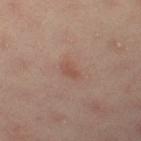The lesion was tiled from a total-body skin photograph and was not biopsied.
The subject is a female aged 53–57.
This is a cross-polarized tile.
A 15 mm close-up tile from a total-body photography series done for melanoma screening.
On the right thigh.
The lesion-visualizer software estimated an outline eccentricity of about 0.8 (0 = round, 1 = elongated) and a symmetry-axis asymmetry near 0.2. And it measured about 6 CIELAB-L* units darker than the surrounding skin. The analysis additionally found a border-irregularity rating of about 2/10. The software also gave a classifier nevus-likeness of about 10/100 and a detector confidence of about 100 out of 100 that the crop contains a lesion.
The recorded lesion diameter is about 2.5 mm.